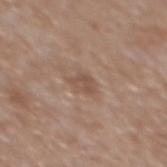The lesion was tiled from a total-body skin photograph and was not biopsied.